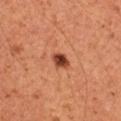notes = catalogued during a skin exam; not biopsied
patient = male, aged approximately 50
anatomic site = the chest
image source = ~15 mm crop, total-body skin-cancer survey